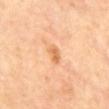Image and clinical context:
Measured at roughly 2.5 mm in maximum diameter. From the mid back. A region of skin cropped from a whole-body photographic capture, roughly 15 mm wide. The total-body-photography lesion software estimated a normalized border contrast of about 6.5.